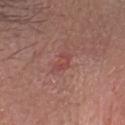Q: Lesion location?
A: the head or neck
Q: Who is the patient?
A: male, approximately 25 years of age
Q: What is the imaging modality?
A: ~15 mm crop, total-body skin-cancer survey
Q: What is the lesion's diameter?
A: about 2.5 mm